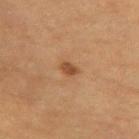Impression:
The lesion was photographed on a routine skin check and not biopsied; there is no pathology result.
Context:
A 15 mm close-up tile from a total-body photography series done for melanoma screening. The tile uses cross-polarized illumination. A female subject approximately 65 years of age. The lesion is on the left thigh.